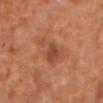Q: Was a biopsy performed?
A: catalogued during a skin exam; not biopsied
Q: What kind of image is this?
A: ~15 mm tile from a whole-body skin photo
Q: Lesion size?
A: about 4.5 mm
Q: Who is the patient?
A: male, approximately 60 years of age
Q: What lighting was used for the tile?
A: cross-polarized
Q: Automated lesion metrics?
A: a footprint of about 10 mm², a shape eccentricity near 0.75, and a shape-asymmetry score of about 0.4 (0 = symmetric)
Q: Where on the body is the lesion?
A: the upper back